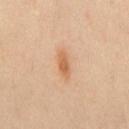Part of a total-body skin-imaging series; this lesion was reviewed on a skin check and was not flagged for biopsy.
A 15 mm crop from a total-body photograph taken for skin-cancer surveillance.
This is a cross-polarized tile.
A male patient, aged approximately 45.
Located on the chest.
Automated tile analysis of the lesion measured a footprint of about 4 mm² and a shape-asymmetry score of about 0.35 (0 = symmetric). The analysis additionally found a lesion–skin lightness drop of about 10 and a lesion-to-skin contrast of about 7.5 (normalized; higher = more distinct). The software also gave a border-irregularity index near 3.5/10, a color-variation rating of about 2.5/10, and a peripheral color-asymmetry measure near 1.